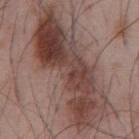Notes:
• biopsy status — catalogued during a skin exam; not biopsied
• diameter — about 14.5 mm
• subject — male, in their mid- to late 50s
• imaging modality — ~15 mm crop, total-body skin-cancer survey
• anatomic site — the abdomen